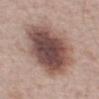This lesion was catalogued during total-body skin photography and was not selected for biopsy. The patient is a male aged 63 to 67. The total-body-photography lesion software estimated a footprint of about 39 mm², an eccentricity of roughly 0.7, and two-axis asymmetry of about 0.2. And it measured an average lesion color of about L≈48 a*≈18 b*≈21 (CIELAB), about 17 CIELAB-L* units darker than the surrounding skin, and a normalized border contrast of about 12. And it measured a detector confidence of about 100 out of 100 that the crop contains a lesion. The lesion is on the chest. This is a white-light tile. Approximately 8.5 mm at its widest. This image is a 15 mm lesion crop taken from a total-body photograph.